A male subject, aged approximately 70; a 15 mm close-up extracted from a 3D total-body photography capture; the lesion is on the chest:
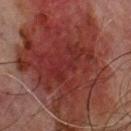diagnosis = an invasive melanoma, superficial spreading type; Breslow depth 0.5 mm, mitotic rate <1/mm²